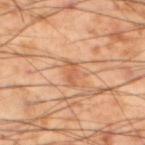Case summary:
• anatomic site — the left lower leg
• subject — male, in their mid- to late 50s
• acquisition — ~15 mm crop, total-body skin-cancer survey
• size — ~3 mm (longest diameter)
• lighting — cross-polarized